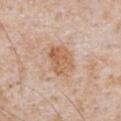<record>
<biopsy_status>not biopsied; imaged during a skin examination</biopsy_status>
<lesion_size>
  <long_diameter_mm_approx>4.0</long_diameter_mm_approx>
</lesion_size>
<automated_metrics>
  <area_mm2_approx>9.5</area_mm2_approx>
  <shape_asymmetry>0.2</shape_asymmetry>
  <border_irregularity_0_10>2.0</border_irregularity_0_10>
  <color_variation_0_10>5.0</color_variation_0_10>
  <peripheral_color_asymmetry>2.0</peripheral_color_asymmetry>
</automated_metrics>
<lighting>white-light</lighting>
<site>front of the torso</site>
<image>
  <source>total-body photography crop</source>
  <field_of_view_mm>15</field_of_view_mm>
</image>
<patient>
  <sex>male</sex>
  <age_approx>65</age_approx>
</patient>
</record>